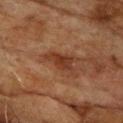Image and clinical context: Approximately 3.5 mm at its widest. The subject is a male aged approximately 75. An algorithmic analysis of the crop reported a detector confidence of about 100 out of 100 that the crop contains a lesion. A 15 mm close-up tile from a total-body photography series done for melanoma screening. Captured under cross-polarized illumination. On the chest.